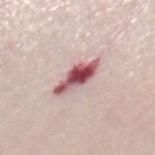<case>
<biopsy_status>not biopsied; imaged during a skin examination</biopsy_status>
<image>
  <source>total-body photography crop</source>
  <field_of_view_mm>15</field_of_view_mm>
</image>
<site>chest</site>
<lesion_size>
  <long_diameter_mm_approx>5.0</long_diameter_mm_approx>
</lesion_size>
<patient>
  <sex>female</sex>
  <age_approx>70</age_approx>
</patient>
</case>